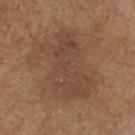  biopsy_status: not biopsied; imaged during a skin examination
  automated_metrics:
    cielab_L: 44
    cielab_a: 18
    cielab_b: 28
    vs_skin_darker_L: 6.0
    border_irregularity_0_10: 4.5
    peripheral_color_asymmetry: 1.0
  lesion_size:
    long_diameter_mm_approx: 8.0
  image:
    source: total-body photography crop
    field_of_view_mm: 15
  lighting: white-light
  patient:
    sex: male
    age_approx: 70
  site: chest